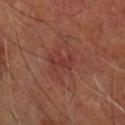<tbp_lesion>
<biopsy_status>not biopsied; imaged during a skin examination</biopsy_status>
</tbp_lesion>